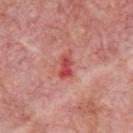Imaged during a routine full-body skin examination; the lesion was not biopsied and no histopathology is available. From the chest. A male patient, aged approximately 75. This is a white-light tile. About 3 mm across. A roughly 15 mm field-of-view crop from a total-body skin photograph.